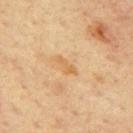Assessment: Imaged during a routine full-body skin examination; the lesion was not biopsied and no histopathology is available. Context: A male subject, about 65 years old. This is a cross-polarized tile. A region of skin cropped from a whole-body photographic capture, roughly 15 mm wide. From the mid back.